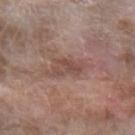Assessment:
The lesion was photographed on a routine skin check and not biopsied; there is no pathology result.
Acquisition and patient details:
A female patient in their mid- to late 70s. Captured under white-light illumination. Approximately 4 mm at its widest. A lesion tile, about 15 mm wide, cut from a 3D total-body photograph. From the arm.